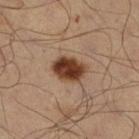Findings:
* follow-up · catalogued during a skin exam; not biopsied
* lighting · cross-polarized illumination
* site · the leg
* lesion diameter · ≈4 mm
* acquisition · 15 mm crop, total-body photography
* subject · male, aged 48 to 52
* automated lesion analysis · lesion-presence confidence of about 100/100